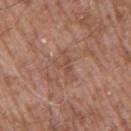No biopsy was performed on this lesion — it was imaged during a full skin examination and was not determined to be concerning.